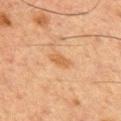Findings:
– notes · catalogued during a skin exam; not biopsied
– automated metrics · a lesion area of about 3 mm², an eccentricity of roughly 0.9, and two-axis asymmetry of about 0.2; a normalized lesion–skin contrast near 6; a within-lesion color-variation index near 1/10 and a peripheral color-asymmetry measure near 0.5; a nevus-likeness score of about 10/100
– lesion size · ≈3 mm
– patient · male, aged approximately 50
– acquisition · ~15 mm crop, total-body skin-cancer survey
– anatomic site · the chest